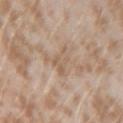Imaged during a routine full-body skin examination; the lesion was not biopsied and no histopathology is available. From the left forearm. A female subject, about 25 years old. A 15 mm crop from a total-body photograph taken for skin-cancer surveillance.